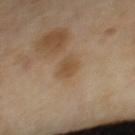  site: upper back
  image:
    source: total-body photography crop
    field_of_view_mm: 15
  automated_metrics:
    lesion_detection_confidence_0_100: 100
  patient:
    sex: female
    age_approx: 45